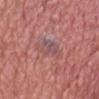Q: Was this lesion biopsied?
A: catalogued during a skin exam; not biopsied
Q: What lighting was used for the tile?
A: white-light
Q: Lesion size?
A: ~3.5 mm (longest diameter)
Q: What is the imaging modality?
A: ~15 mm crop, total-body skin-cancer survey
Q: What did automated image analysis measure?
A: a footprint of about 7.5 mm² and a shape-asymmetry score of about 0.4 (0 = symmetric); an average lesion color of about L≈51 a*≈23 b*≈19 (CIELAB), roughly 6 lightness units darker than nearby skin, and a normalized lesion–skin contrast near 5.5; a classifier nevus-likeness of about 0/100 and a lesion-detection confidence of about 95/100
Q: Patient demographics?
A: male, aged around 60
Q: Lesion location?
A: the head or neck